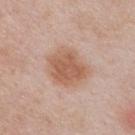<tbp_lesion>
<automated_metrics>
  <area_mm2_approx>17.0</area_mm2_approx>
  <shape_asymmetry>0.15</shape_asymmetry>
  <cielab_L>59</cielab_L>
  <cielab_a>20</cielab_a>
  <cielab_b>30</cielab_b>
  <vs_skin_darker_L>10.0</vs_skin_darker_L>
  <vs_skin_contrast_norm>7.5</vs_skin_contrast_norm>
  <color_variation_0_10>3.5</color_variation_0_10>
  <peripheral_color_asymmetry>1.0</peripheral_color_asymmetry>
</automated_metrics>
<patient>
  <sex>male</sex>
  <age_approx>50</age_approx>
</patient>
<image>
  <source>total-body photography crop</source>
  <field_of_view_mm>15</field_of_view_mm>
</image>
<site>chest</site>
<lighting>white-light</lighting>
</tbp_lesion>